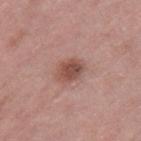Q: Is there a histopathology result?
A: catalogued during a skin exam; not biopsied
Q: Where on the body is the lesion?
A: the right thigh
Q: How large is the lesion?
A: ~3.5 mm (longest diameter)
Q: Who is the patient?
A: female, roughly 70 years of age
Q: How was this image acquired?
A: total-body-photography crop, ~15 mm field of view